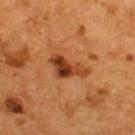| feature | finding |
|---|---|
| notes | no biopsy performed (imaged during a skin exam) |
| patient | male, in their mid- to late 50s |
| imaging modality | total-body-photography crop, ~15 mm field of view |
| lesion size | ≈5 mm |
| body site | the upper back |
| automated lesion analysis | a lesion area of about 8.5 mm², an eccentricity of roughly 0.85, and a symmetry-axis asymmetry near 0.45; a lesion color around L≈38 a*≈26 b*≈36 in CIELAB, roughly 13 lightness units darker than nearby skin, and a lesion-to-skin contrast of about 10.5 (normalized; higher = more distinct); a border-irregularity rating of about 5/10, internal color variation of about 8.5 on a 0–10 scale, and a peripheral color-asymmetry measure near 3.5 |